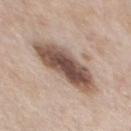biopsy status = imaged on a skin check; not biopsied | lighting = white-light | image source = ~15 mm crop, total-body skin-cancer survey | body site = the chest | subject = male, aged around 60 | size = about 7.5 mm.